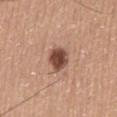Case summary:
- site — the mid back
- image — ~15 mm tile from a whole-body skin photo
- subject — male, aged 58–62
- tile lighting — white-light